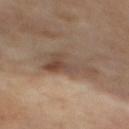The lesion was tiled from a total-body skin photograph and was not biopsied. From the mid back. The recorded lesion diameter is about 5 mm. This is a cross-polarized tile. The lesion-visualizer software estimated a detector confidence of about 100 out of 100 that the crop contains a lesion. A 15 mm crop from a total-body photograph taken for skin-cancer surveillance.